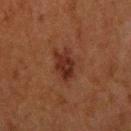Findings:
– workup: total-body-photography surveillance lesion; no biopsy
– subject: male, approximately 50 years of age
– size: about 4 mm
– acquisition: 15 mm crop, total-body photography
– body site: the head or neck
– image-analysis metrics: a classifier nevus-likeness of about 40/100 and lesion-presence confidence of about 100/100